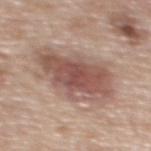Impression: The lesion was photographed on a routine skin check and not biopsied; there is no pathology result. Image and clinical context: A 15 mm crop from a total-body photograph taken for skin-cancer surveillance. The subject is a female aged 58 to 62. Located on the upper back. This is a white-light tile.